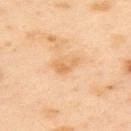Captured during whole-body skin photography for melanoma surveillance; the lesion was not biopsied. The recorded lesion diameter is about 3 mm. A female patient roughly 40 years of age. A roughly 15 mm field-of-view crop from a total-body skin photograph. From the back. The tile uses cross-polarized illumination.